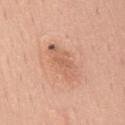Notes:
• follow-up — total-body-photography surveillance lesion; no biopsy
• lesion size — about 5 mm
• subject — female, in their 50s
• location — the mid back
• TBP lesion metrics — a mean CIELAB color near L≈62 a*≈23 b*≈32 and about 9 CIELAB-L* units darker than the surrounding skin; an automated nevus-likeness rating near 0 out of 100 and lesion-presence confidence of about 100/100
• image source — ~15 mm tile from a whole-body skin photo
• illumination — white-light